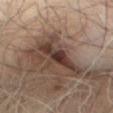Captured during whole-body skin photography for melanoma surveillance; the lesion was not biopsied.
This image is a 15 mm lesion crop taken from a total-body photograph.
Located on the mid back.
Longest diameter approximately 6.5 mm.
The lesion-visualizer software estimated a lesion area of about 13 mm², a shape eccentricity near 0.9, and a shape-asymmetry score of about 0.5 (0 = symmetric). The software also gave a classifier nevus-likeness of about 0/100 and lesion-presence confidence of about 70/100.
A male subject aged around 60.
Imaged with cross-polarized lighting.